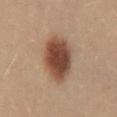Findings:
- notes — no biopsy performed (imaged during a skin exam)
- site — the lower back
- size — ≈6.5 mm
- acquisition — ~15 mm tile from a whole-body skin photo
- subject — female, aged 23 to 27
- lighting — white-light illumination
- automated metrics — a lesion area of about 20 mm², a shape eccentricity near 0.8, and two-axis asymmetry of about 0.1; an average lesion color of about L≈48 a*≈20 b*≈29 (CIELAB) and a normalized lesion–skin contrast near 11.5